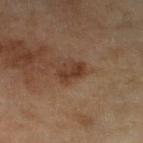{"biopsy_status": "not biopsied; imaged during a skin examination", "image": {"source": "total-body photography crop", "field_of_view_mm": 15}, "patient": {"sex": "female", "age_approx": 60}, "lighting": "cross-polarized", "lesion_size": {"long_diameter_mm_approx": 3.0}, "site": "left lower leg"}